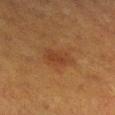Q: Was a biopsy performed?
A: total-body-photography surveillance lesion; no biopsy
Q: What is the imaging modality?
A: ~15 mm tile from a whole-body skin photo
Q: Who is the patient?
A: female, aged 53 to 57
Q: Where on the body is the lesion?
A: the right lower leg